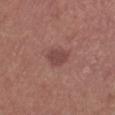Q: What did automated image analysis measure?
A: a lesion color around L≈44 a*≈22 b*≈22 in CIELAB, about 8 CIELAB-L* units darker than the surrounding skin, and a normalized border contrast of about 6.5
Q: What lighting was used for the tile?
A: white-light illumination
Q: What is the lesion's diameter?
A: ≈3 mm
Q: Who is the patient?
A: male, aged 68 to 72
Q: Lesion location?
A: the right lower leg
Q: What kind of image is this?
A: ~15 mm crop, total-body skin-cancer survey